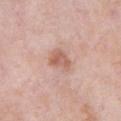Captured during whole-body skin photography for melanoma surveillance; the lesion was not biopsied. This is a white-light tile. The patient is a male about 55 years old. The lesion's longest dimension is about 3 mm. The total-body-photography lesion software estimated a footprint of about 6 mm², a shape eccentricity near 0.7, and a symmetry-axis asymmetry near 0.25. A 15 mm close-up tile from a total-body photography series done for melanoma screening. From the abdomen.